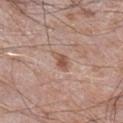Clinical impression:
The lesion was photographed on a routine skin check and not biopsied; there is no pathology result.
Context:
A male subject approximately 60 years of age. The lesion's longest dimension is about 3 mm. A region of skin cropped from a whole-body photographic capture, roughly 15 mm wide. From the right lower leg. The tile uses white-light illumination.